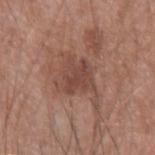Clinical impression: Recorded during total-body skin imaging; not selected for excision or biopsy. Background: The lesion is located on the left forearm. A roughly 15 mm field-of-view crop from a total-body skin photograph. The patient is a male aged around 55. Automated image analysis of the tile measured an area of roughly 8.5 mm², an eccentricity of roughly 0.5, and a symmetry-axis asymmetry near 0.25. The analysis additionally found a border-irregularity rating of about 4/10, a color-variation rating of about 2.5/10, and a peripheral color-asymmetry measure near 1. And it measured a nevus-likeness score of about 0/100 and a detector confidence of about 100 out of 100 that the crop contains a lesion. The tile uses white-light illumination.